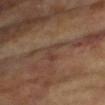On the left upper arm.
Captured under cross-polarized illumination.
The patient is a female roughly 75 years of age.
This image is a 15 mm lesion crop taken from a total-body photograph.